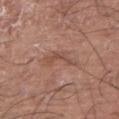notes: no biopsy performed (imaged during a skin exam); anatomic site: the right upper arm; subject: male, roughly 75 years of age; imaging modality: ~15 mm crop, total-body skin-cancer survey; tile lighting: white-light illumination; size: ~3.5 mm (longest diameter).